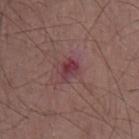The lesion was photographed on a routine skin check and not biopsied; there is no pathology result. A male subject approximately 60 years of age. The lesion is on the chest. A 15 mm close-up extracted from a 3D total-body photography capture. Longest diameter approximately 2.5 mm.